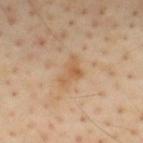| field | value |
|---|---|
| biopsy status | no biopsy performed (imaged during a skin exam) |
| location | the upper back |
| imaging modality | ~15 mm tile from a whole-body skin photo |
| patient | male, about 55 years old |